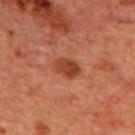  biopsy_status: not biopsied; imaged during a skin examination
  site: upper back
  patient:
    sex: male
    age_approx: 70
  image:
    source: total-body photography crop
    field_of_view_mm: 15